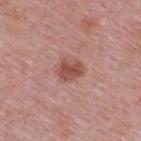Assessment:
No biopsy was performed on this lesion — it was imaged during a full skin examination and was not determined to be concerning.
Context:
The lesion is on the upper back. Cropped from a whole-body photographic skin survey; the tile spans about 15 mm. Approximately 3 mm at its widest. Captured under white-light illumination. A male patient, roughly 55 years of age.